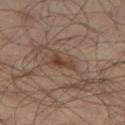  biopsy_status: not biopsied; imaged during a skin examination
  patient:
    sex: male
    age_approx: 65
  site: left thigh
  image:
    source: total-body photography crop
    field_of_view_mm: 15
  lighting: cross-polarized